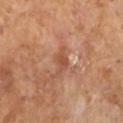Impression:
The lesion was photographed on a routine skin check and not biopsied; there is no pathology result.
Background:
A male patient, aged 63–67. Longest diameter approximately 3 mm. The total-body-photography lesion software estimated an eccentricity of roughly 0.8 and two-axis asymmetry of about 0.3. It also reported a mean CIELAB color near L≈49 a*≈23 b*≈31, roughly 8 lightness units darker than nearby skin, and a lesion-to-skin contrast of about 6 (normalized; higher = more distinct). And it measured a border-irregularity index near 3.5/10, a within-lesion color-variation index near 2.5/10, and a peripheral color-asymmetry measure near 1. The software also gave a classifier nevus-likeness of about 0/100. This is a cross-polarized tile. A close-up tile cropped from a whole-body skin photograph, about 15 mm across.